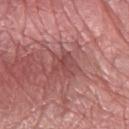The lesion was tiled from a total-body skin photograph and was not biopsied.
The recorded lesion diameter is about 3 mm.
Captured under white-light illumination.
A roughly 15 mm field-of-view crop from a total-body skin photograph.
The subject is a female approximately 60 years of age.
An algorithmic analysis of the crop reported a mean CIELAB color near L≈47 a*≈27 b*≈22 and about 7 CIELAB-L* units darker than the surrounding skin. And it measured a border-irregularity rating of about 3.5/10 and peripheral color asymmetry of about 1. The software also gave lesion-presence confidence of about 85/100.
From the right forearm.